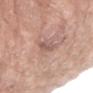| key | value |
|---|---|
| workup | total-body-photography surveillance lesion; no biopsy |
| imaging modality | total-body-photography crop, ~15 mm field of view |
| diameter | about 3 mm |
| lighting | white-light illumination |
| subject | female, about 75 years old |
| body site | the left forearm |